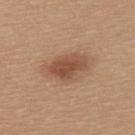Q: Was a biopsy performed?
A: no biopsy performed (imaged during a skin exam)
Q: What kind of image is this?
A: total-body-photography crop, ~15 mm field of view
Q: Patient demographics?
A: female, approximately 30 years of age
Q: Where on the body is the lesion?
A: the upper back